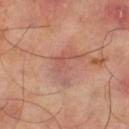The lesion was tiled from a total-body skin photograph and was not biopsied.
This is a cross-polarized tile.
Cropped from a whole-body photographic skin survey; the tile spans about 15 mm.
Located on the right thigh.
The lesion's longest dimension is about 4 mm.